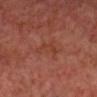workup: total-body-photography surveillance lesion; no biopsy
acquisition: 15 mm crop, total-body photography
body site: the head or neck
lesion diameter: about 3.5 mm
automated metrics: an outline eccentricity of about 0.9 (0 = round, 1 = elongated); a mean CIELAB color near L≈37 a*≈25 b*≈29 and roughly 4 lightness units darker than nearby skin; a border-irregularity index near 6.5/10 and a color-variation rating of about 1/10
lighting: cross-polarized illumination
subject: male, about 60 years old